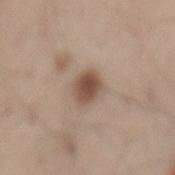The lesion was photographed on a routine skin check and not biopsied; there is no pathology result.
This is a white-light tile.
Located on the mid back.
The subject is a male aged around 55.
A region of skin cropped from a whole-body photographic capture, roughly 15 mm wide.